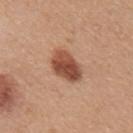Findings:
• site · the right upper arm
• imaging modality · total-body-photography crop, ~15 mm field of view
• TBP lesion metrics · a mean CIELAB color near L≈49 a*≈24 b*≈31, a lesion–skin lightness drop of about 15, and a normalized lesion–skin contrast near 10.5; a border-irregularity index near 2/10 and a within-lesion color-variation index near 5.5/10; lesion-presence confidence of about 100/100
• tile lighting · white-light
• subject · female, about 45 years old